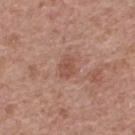  biopsy_status: not biopsied; imaged during a skin examination
  lesion_size:
    long_diameter_mm_approx: 2.5
  patient:
    sex: female
    age_approx: 65
  image:
    source: total-body photography crop
    field_of_view_mm: 15
  lighting: white-light
  site: upper back